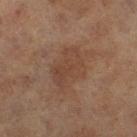Assessment: No biopsy was performed on this lesion — it was imaged during a full skin examination and was not determined to be concerning. Acquisition and patient details: The tile uses cross-polarized illumination. A close-up tile cropped from a whole-body skin photograph, about 15 mm across. A female patient, in their 60s. The lesion is located on the left thigh. Automated tile analysis of the lesion measured an area of roughly 12 mm² and a shape eccentricity near 0.75. It also reported about 5 CIELAB-L* units darker than the surrounding skin and a normalized border contrast of about 5.5. The analysis additionally found a lesion-detection confidence of about 100/100.